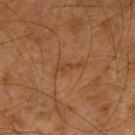Part of a total-body skin-imaging series; this lesion was reviewed on a skin check and was not flagged for biopsy. Cropped from a total-body skin-imaging series; the visible field is about 15 mm. Captured under cross-polarized illumination. The lesion's longest dimension is about 3 mm. The lesion-visualizer software estimated a lesion area of about 3 mm², a shape eccentricity near 0.95, and two-axis asymmetry of about 0.45. The analysis additionally found a border-irregularity rating of about 5/10, a color-variation rating of about 0/10, and a peripheral color-asymmetry measure near 0. A male subject approximately 65 years of age. Located on the left lower leg.